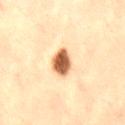The lesion was tiled from a total-body skin photograph and was not biopsied. The lesion is located on the lower back. A close-up tile cropped from a whole-body skin photograph, about 15 mm across. A female patient, approximately 45 years of age.